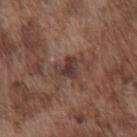Background: The patient is a male aged 73 to 77. Cropped from a total-body skin-imaging series; the visible field is about 15 mm. The tile uses white-light illumination. About 3 mm across. Located on the chest. The lesion-visualizer software estimated roughly 9 lightness units darker than nearby skin.